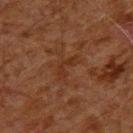Clinical impression:
Captured during whole-body skin photography for melanoma surveillance; the lesion was not biopsied.
Background:
The lesion is on the upper back. A male subject, approximately 60 years of age. A close-up tile cropped from a whole-body skin photograph, about 15 mm across. The lesion-visualizer software estimated an average lesion color of about L≈24 a*≈18 b*≈25 (CIELAB), a lesion–skin lightness drop of about 4, and a normalized lesion–skin contrast near 5.5. The software also gave a border-irregularity rating of about 6.5/10, internal color variation of about 0 on a 0–10 scale, and radial color variation of about 0. Captured under cross-polarized illumination. Approximately 3.5 mm at its widest.